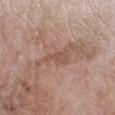| feature | finding |
|---|---|
| biopsy status | catalogued during a skin exam; not biopsied |
| tile lighting | white-light |
| lesion diameter | ≈3.5 mm |
| acquisition | total-body-photography crop, ~15 mm field of view |
| body site | the arm |
| patient | female, aged around 75 |